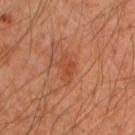Assessment:
Part of a total-body skin-imaging series; this lesion was reviewed on a skin check and was not flagged for biopsy.
Image and clinical context:
The subject is a male approximately 45 years of age. Approximately 3 mm at its widest. An algorithmic analysis of the crop reported a footprint of about 4 mm². It also reported a border-irregularity rating of about 3.5/10 and a within-lesion color-variation index near 2/10. And it measured a nevus-likeness score of about 10/100 and a lesion-detection confidence of about 100/100. The lesion is on the left upper arm. Imaged with cross-polarized lighting. A lesion tile, about 15 mm wide, cut from a 3D total-body photograph.